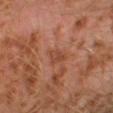{"biopsy_status": "not biopsied; imaged during a skin examination", "lighting": "cross-polarized", "image": {"source": "total-body photography crop", "field_of_view_mm": 15}, "lesion_size": {"long_diameter_mm_approx": 2.5}, "automated_metrics": {"area_mm2_approx": 3.0, "eccentricity": 0.8, "shape_asymmetry": 0.4}, "patient": {"sex": "male", "age_approx": 30}, "site": "leg"}